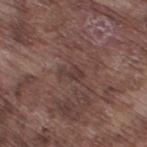subject: male, approximately 75 years of age; imaging modality: ~15 mm crop, total-body skin-cancer survey; site: the right thigh; size: ~2.5 mm (longest diameter); illumination: white-light.